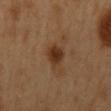The lesion was tiled from a total-body skin photograph and was not biopsied. Located on the mid back. A male subject approximately 50 years of age. A 15 mm close-up tile from a total-body photography series done for melanoma screening.